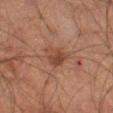Assessment:
The lesion was photographed on a routine skin check and not biopsied; there is no pathology result.
Acquisition and patient details:
A lesion tile, about 15 mm wide, cut from a 3D total-body photograph. A male subject roughly 55 years of age. Approximately 3.5 mm at its widest. The lesion is on the left thigh.